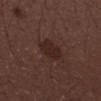Assessment: Part of a total-body skin-imaging series; this lesion was reviewed on a skin check and was not flagged for biopsy. Background: A 15 mm close-up extracted from a 3D total-body photography capture. The lesion is located on the left forearm. The tile uses white-light illumination. A male subject, about 30 years old.